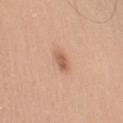follow-up: imaged on a skin check; not biopsied | automated metrics: an area of roughly 3.5 mm² and two-axis asymmetry of about 0.2; about 10 CIELAB-L* units darker than the surrounding skin and a normalized lesion–skin contrast near 7; a border-irregularity index near 2/10, internal color variation of about 2.5 on a 0–10 scale, and peripheral color asymmetry of about 1 | body site: the arm | imaging modality: ~15 mm tile from a whole-body skin photo | illumination: white-light illumination | subject: male, aged 58–62 | lesion size: ≈2.5 mm.